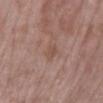Captured during whole-body skin photography for melanoma surveillance; the lesion was not biopsied.
This is a white-light tile.
A 15 mm close-up tile from a total-body photography series done for melanoma screening.
A female patient, in their 70s.
Longest diameter approximately 2.5 mm.
The lesion is on the right lower leg.